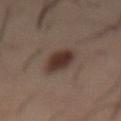Notes:
- notes: total-body-photography surveillance lesion; no biopsy
- site: the abdomen
- image source: total-body-photography crop, ~15 mm field of view
- patient: male, in their mid- to late 50s